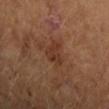<case>
<biopsy_status>not biopsied; imaged during a skin examination</biopsy_status>
<lighting>cross-polarized</lighting>
<image>
  <source>total-body photography crop</source>
  <field_of_view_mm>15</field_of_view_mm>
</image>
<patient>
  <sex>female</sex>
  <age_approx>60</age_approx>
</patient>
<site>arm</site>
<automated_metrics>
  <vs_skin_darker_L>7.0</vs_skin_darker_L>
  <vs_skin_contrast_norm>6.5</vs_skin_contrast_norm>
  <border_irregularity_0_10>4.0</border_irregularity_0_10>
  <color_variation_0_10>3.0</color_variation_0_10>
  <peripheral_color_asymmetry>1.0</peripheral_color_asymmetry>
  <nevus_likeness_0_100>0</nevus_likeness_0_100>
  <lesion_detection_confidence_0_100>100</lesion_detection_confidence_0_100>
</automated_metrics>
</case>